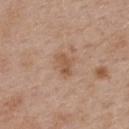subject = female, roughly 40 years of age | body site = the upper back | diameter = about 3 mm | TBP lesion metrics = an area of roughly 4.5 mm² and two-axis asymmetry of about 0.3; internal color variation of about 3 on a 0–10 scale and peripheral color asymmetry of about 1.5 | image source = ~15 mm tile from a whole-body skin photo.